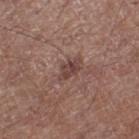Assessment: Imaged during a routine full-body skin examination; the lesion was not biopsied and no histopathology is available. Image and clinical context: Imaged with white-light lighting. The total-body-photography lesion software estimated an area of roughly 4 mm², an eccentricity of roughly 0.8, and two-axis asymmetry of about 0.3. The analysis additionally found a nevus-likeness score of about 5/100 and a detector confidence of about 100 out of 100 that the crop contains a lesion. A 15 mm close-up tile from a total-body photography series done for melanoma screening. Located on the right lower leg. A male patient, aged around 70.